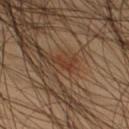Clinical impression: This lesion was catalogued during total-body skin photography and was not selected for biopsy. Background: This is a cross-polarized tile. A region of skin cropped from a whole-body photographic capture, roughly 15 mm wide. Approximately 2 mm at its widest. On the right thigh. The subject is a male aged 43–47.